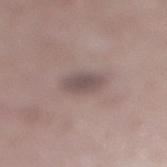- workup · total-body-photography surveillance lesion; no biopsy
- acquisition · total-body-photography crop, ~15 mm field of view
- site · the right lower leg
- patient · female, aged 38 to 42